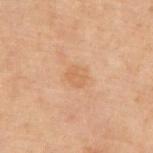Clinical impression:
No biopsy was performed on this lesion — it was imaged during a full skin examination and was not determined to be concerning.
Background:
Located on the chest. This is a cross-polarized tile. Measured at roughly 2.5 mm in maximum diameter. A male patient, roughly 70 years of age. Cropped from a total-body skin-imaging series; the visible field is about 15 mm.